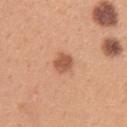Clinical summary: A female patient, aged 28–32. The lesion is on the right upper arm. This image is a 15 mm lesion crop taken from a total-body photograph.